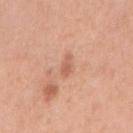notes: total-body-photography surveillance lesion; no biopsy | image source: 15 mm crop, total-body photography | location: the right upper arm | diameter: about 2.5 mm | tile lighting: white-light | patient: female, aged approximately 55.